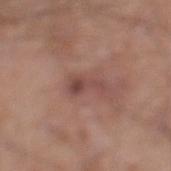{
  "image": {
    "source": "total-body photography crop",
    "field_of_view_mm": 15
  },
  "lesion_size": {
    "long_diameter_mm_approx": 4.5
  },
  "patient": {
    "sex": "male",
    "age_approx": 20
  },
  "site": "left lower leg",
  "lighting": "white-light"
}